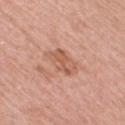– biopsy status — imaged on a skin check; not biopsied